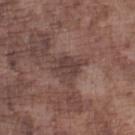Assessment:
Part of a total-body skin-imaging series; this lesion was reviewed on a skin check and was not flagged for biopsy.
Acquisition and patient details:
A male patient aged around 75. A lesion tile, about 15 mm wide, cut from a 3D total-body photograph. Located on the left lower leg.